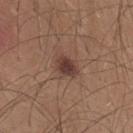<record>
  <lesion_size>
    <long_diameter_mm_approx>3.0</long_diameter_mm_approx>
  </lesion_size>
  <site>back</site>
  <image>
    <source>total-body photography crop</source>
    <field_of_view_mm>15</field_of_view_mm>
  </image>
  <patient>
    <sex>male</sex>
    <age_approx>25</age_approx>
  </patient>
</record>